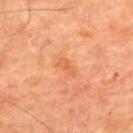biopsy_status: not biopsied; imaged during a skin examination
patient:
  sex: male
  age_approx: 65
lesion_size:
  long_diameter_mm_approx: 2.5
image:
  source: total-body photography crop
  field_of_view_mm: 15
site: upper back
lighting: cross-polarized